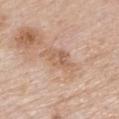This lesion was catalogued during total-body skin photography and was not selected for biopsy.
A lesion tile, about 15 mm wide, cut from a 3D total-body photograph.
Located on the mid back.
Measured at roughly 5.5 mm in maximum diameter.
A male subject aged approximately 85 or older.
The tile uses white-light illumination.
An algorithmic analysis of the crop reported a lesion area of about 9 mm² and two-axis asymmetry of about 0.3. The analysis additionally found an average lesion color of about L≈61 a*≈18 b*≈31 (CIELAB) and roughly 8 lightness units darker than nearby skin.